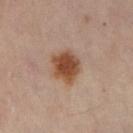No biopsy was performed on this lesion — it was imaged during a full skin examination and was not determined to be concerning. Located on the left lower leg. A 15 mm close-up extracted from a 3D total-body photography capture. Automated tile analysis of the lesion measured a lesion area of about 11 mm² and a shape-asymmetry score of about 0.2 (0 = symmetric). It also reported an average lesion color of about L≈48 a*≈20 b*≈31 (CIELAB), a lesion–skin lightness drop of about 13, and a lesion-to-skin contrast of about 10.5 (normalized; higher = more distinct). The analysis additionally found a within-lesion color-variation index near 4.5/10 and a peripheral color-asymmetry measure near 1. The software also gave an automated nevus-likeness rating near 100 out of 100 and a detector confidence of about 100 out of 100 that the crop contains a lesion. Measured at roughly 4 mm in maximum diameter. Imaged with cross-polarized lighting. A female subject, aged approximately 60.